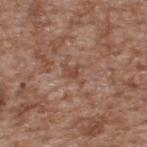Part of a total-body skin-imaging series; this lesion was reviewed on a skin check and was not flagged for biopsy.
From the upper back.
Captured under white-light illumination.
The recorded lesion diameter is about 3 mm.
The subject is a male approximately 65 years of age.
A close-up tile cropped from a whole-body skin photograph, about 15 mm across.